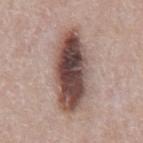Q: Lesion size?
A: ≈9.5 mm
Q: How was the tile lit?
A: white-light illumination
Q: What is the imaging modality?
A: 15 mm crop, total-body photography
Q: What is the anatomic site?
A: the chest
Q: What did automated image analysis measure?
A: a footprint of about 29 mm², a shape eccentricity near 0.9, and a shape-asymmetry score of about 0.15 (0 = symmetric); an average lesion color of about L≈47 a*≈17 b*≈21 (CIELAB) and a lesion-to-skin contrast of about 13 (normalized; higher = more distinct); a border-irregularity rating of about 2.5/10, internal color variation of about 10 on a 0–10 scale, and peripheral color asymmetry of about 3.5; a nevus-likeness score of about 100/100 and a lesion-detection confidence of about 100/100
Q: What are the patient's age and sex?
A: male, aged 68–72